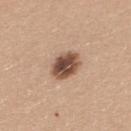size: ~3.5 mm (longest diameter) | tile lighting: white-light | site: the back | patient: female, approximately 25 years of age | image: ~15 mm crop, total-body skin-cancer survey | automated lesion analysis: a lesion color around L≈50 a*≈19 b*≈28 in CIELAB, a lesion–skin lightness drop of about 18, and a normalized lesion–skin contrast near 12.